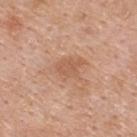notes: no biopsy performed (imaged during a skin exam)
illumination: white-light
patient: female, aged approximately 40
location: the upper back
image: ~15 mm tile from a whole-body skin photo
image-analysis metrics: border irregularity of about 3.5 on a 0–10 scale and a peripheral color-asymmetry measure near 0.5; a classifier nevus-likeness of about 0/100 and lesion-presence confidence of about 100/100
diameter: about 3 mm